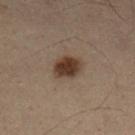Imaged during a routine full-body skin examination; the lesion was not biopsied and no histopathology is available. A male patient, aged approximately 55. Cropped from a total-body skin-imaging series; the visible field is about 15 mm. The lesion is on the left lower leg.